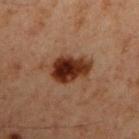{
  "biopsy_status": "not biopsied; imaged during a skin examination",
  "site": "left upper arm",
  "lesion_size": {
    "long_diameter_mm_approx": 5.0
  },
  "automated_metrics": {
    "area_mm2_approx": 11.0,
    "shape_asymmetry": 0.25,
    "cielab_L": 23,
    "cielab_a": 19,
    "cielab_b": 23,
    "vs_skin_darker_L": 14.0,
    "vs_skin_contrast_norm": 14.0,
    "nevus_likeness_0_100": 100,
    "lesion_detection_confidence_0_100": 100
  },
  "patient": {
    "sex": "male",
    "age_approx": 60
  },
  "lighting": "cross-polarized",
  "image": {
    "source": "total-body photography crop",
    "field_of_view_mm": 15
  }
}